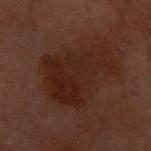Impression:
The lesion was photographed on a routine skin check and not biopsied; there is no pathology result.
Clinical summary:
Cropped from a whole-body photographic skin survey; the tile spans about 15 mm. The lesion is on the front of the torso. About 7.5 mm across. Imaged with cross-polarized lighting. The total-body-photography lesion software estimated a lesion color around L≈19 a*≈17 b*≈21 in CIELAB, roughly 5 lightness units darker than nearby skin, and a normalized lesion–skin contrast near 7. The subject is a female in their 60s.